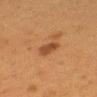Image and clinical context:
The lesion's longest dimension is about 3 mm. Imaged with cross-polarized lighting. Located on the mid back. A female patient in their 50s. A close-up tile cropped from a whole-body skin photograph, about 15 mm across.